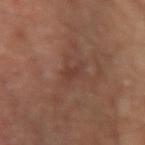No biopsy was performed on this lesion — it was imaged during a full skin examination and was not determined to be concerning.
A male patient, aged around 65.
On the right upper arm.
Longest diameter approximately 2.5 mm.
A lesion tile, about 15 mm wide, cut from a 3D total-body photograph.